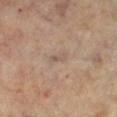Q: Is there a histopathology result?
A: total-body-photography surveillance lesion; no biopsy
Q: How was this image acquired?
A: ~15 mm crop, total-body skin-cancer survey
Q: What is the anatomic site?
A: the left lower leg
Q: Patient demographics?
A: female, approximately 60 years of age
Q: What is the lesion's diameter?
A: ≈2.5 mm
Q: How was the tile lit?
A: cross-polarized
Q: Automated lesion metrics?
A: an area of roughly 2.5 mm² and an eccentricity of roughly 0.95